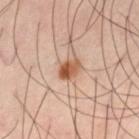biopsy status: total-body-photography surveillance lesion; no biopsy | diameter: ≈3 mm | patient: male, aged approximately 40 | image: total-body-photography crop, ~15 mm field of view | anatomic site: the left thigh | automated lesion analysis: an area of roughly 4.5 mm², an eccentricity of roughly 0.75, and a symmetry-axis asymmetry near 0.15; a mean CIELAB color near L≈54 a*≈23 b*≈34 and a normalized border contrast of about 10.5; a nevus-likeness score of about 95/100 and a lesion-detection confidence of about 100/100.